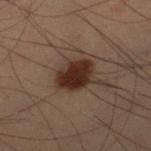The lesion was tiled from a total-body skin photograph and was not biopsied. The subject is a male aged approximately 55. A 15 mm close-up extracted from a 3D total-body photography capture. Located on the leg.